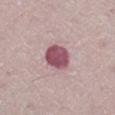The lesion was tiled from a total-body skin photograph and was not biopsied.
The recorded lesion diameter is about 3.5 mm.
A female subject aged approximately 55.
This image is a 15 mm lesion crop taken from a total-body photograph.
From the right lower leg.
Imaged with white-light lighting.